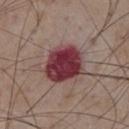* workup: imaged on a skin check; not biopsied
* patient: male, aged 73–77
* location: the chest
* automated metrics: a classifier nevus-likeness of about 0/100 and a lesion-detection confidence of about 100/100
* acquisition: total-body-photography crop, ~15 mm field of view